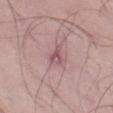A 15 mm close-up tile from a total-body photography series done for melanoma screening. The lesion is located on the left thigh. A male subject, about 50 years old.